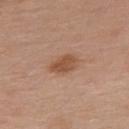Clinical impression:
Captured during whole-body skin photography for melanoma surveillance; the lesion was not biopsied.